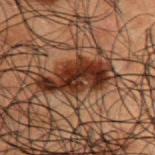The lesion was photographed on a routine skin check and not biopsied; there is no pathology result. A male subject, aged 48–52. Captured under cross-polarized illumination. This image is a 15 mm lesion crop taken from a total-body photograph. On the upper back. The lesion's longest dimension is about 8 mm.